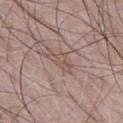<tbp_lesion>
<biopsy_status>not biopsied; imaged during a skin examination</biopsy_status>
<automated_metrics>
  <border_irregularity_0_10>4.5</border_irregularity_0_10>
  <color_variation_0_10>3.0</color_variation_0_10>
  <peripheral_color_asymmetry>1.5</peripheral_color_asymmetry>
  <lesion_detection_confidence_0_100>60</lesion_detection_confidence_0_100>
</automated_metrics>
<image>
  <source>total-body photography crop</source>
  <field_of_view_mm>15</field_of_view_mm>
</image>
<patient>
  <sex>male</sex>
  <age_approx>45</age_approx>
</patient>
<lighting>white-light</lighting>
<site>chest</site>
<lesion_size>
  <long_diameter_mm_approx>3.5</long_diameter_mm_approx>
</lesion_size>
</tbp_lesion>